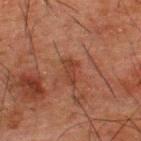Clinical impression: Recorded during total-body skin imaging; not selected for excision or biopsy. Background: The lesion is located on the upper back. A close-up tile cropped from a whole-body skin photograph, about 15 mm across. The tile uses cross-polarized illumination. About 3 mm across. A male patient, aged around 50.